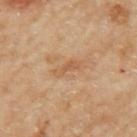biopsy status=catalogued during a skin exam; not biopsied
lighting=cross-polarized
image source=~15 mm tile from a whole-body skin photo
lesion diameter=~3 mm (longest diameter)
TBP lesion metrics=a footprint of about 3.5 mm² and two-axis asymmetry of about 0.4; about 7 CIELAB-L* units darker than the surrounding skin
subject=female, aged 58 to 62
location=the left upper arm